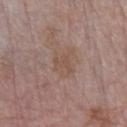The lesion was photographed on a routine skin check and not biopsied; there is no pathology result.
The tile uses white-light illumination.
The total-body-photography lesion software estimated a lesion area of about 6 mm², a shape eccentricity near 0.7, and two-axis asymmetry of about 0.4.
A lesion tile, about 15 mm wide, cut from a 3D total-body photograph.
The subject is a female in their mid-60s.
The lesion is on the left forearm.